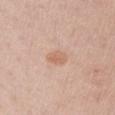Notes:
• subject · male, about 60 years old
• automated metrics · a lesion area of about 4 mm², an eccentricity of roughly 0.8, and two-axis asymmetry of about 0.15; a mean CIELAB color near L≈64 a*≈20 b*≈31, about 8 CIELAB-L* units darker than the surrounding skin, and a normalized lesion–skin contrast near 5.5; border irregularity of about 1.5 on a 0–10 scale and peripheral color asymmetry of about 1; a nevus-likeness score of about 10/100 and a lesion-detection confidence of about 100/100
• image source · total-body-photography crop, ~15 mm field of view
• anatomic site · the left upper arm
• lesion diameter · ≈3 mm